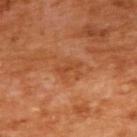Located on the upper back. Cropped from a total-body skin-imaging series; the visible field is about 15 mm. Measured at roughly 3 mm in maximum diameter. The patient is a female about 55 years old. This is a cross-polarized tile.